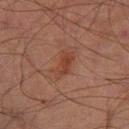{
  "biopsy_status": "not biopsied; imaged during a skin examination",
  "patient": {
    "sex": "male",
    "age_approx": 70
  },
  "image": {
    "source": "total-body photography crop",
    "field_of_view_mm": 15
  },
  "automated_metrics": {
    "cielab_L": 33,
    "cielab_a": 20,
    "cielab_b": 25,
    "vs_skin_darker_L": 6.0,
    "vs_skin_contrast_norm": 6.5,
    "border_irregularity_0_10": 3.5,
    "color_variation_0_10": 1.5,
    "peripheral_color_asymmetry": 0.5,
    "lesion_detection_confidence_0_100": 100
  },
  "lesion_size": {
    "long_diameter_mm_approx": 3.5
  },
  "lighting": "cross-polarized",
  "site": "right lower leg"
}